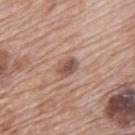{
  "biopsy_status": "not biopsied; imaged during a skin examination",
  "lesion_size": {
    "long_diameter_mm_approx": 3.0
  },
  "site": "mid back",
  "lighting": "white-light",
  "image": {
    "source": "total-body photography crop",
    "field_of_view_mm": 15
  },
  "patient": {
    "sex": "male",
    "age_approx": 70
  }
}